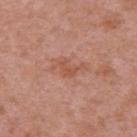Captured during whole-body skin photography for melanoma surveillance; the lesion was not biopsied. Approximately 3.5 mm at its widest. The tile uses white-light illumination. Cropped from a total-body skin-imaging series; the visible field is about 15 mm. A female patient aged around 40. Located on the left upper arm.